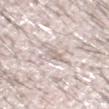Measured at roughly 3 mm in maximum diameter. Automated tile analysis of the lesion measured an average lesion color of about L≈69 a*≈11 b*≈20 (CIELAB), roughly 9 lightness units darker than nearby skin, and a normalized border contrast of about 5. And it measured border irregularity of about 3 on a 0–10 scale, internal color variation of about 0 on a 0–10 scale, and peripheral color asymmetry of about 0. A 15 mm crop from a total-body photograph taken for skin-cancer surveillance. The lesion is located on the head or neck. A male subject, about 60 years old.